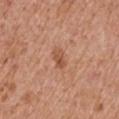The lesion was photographed on a routine skin check and not biopsied; there is no pathology result.
Approximately 2.5 mm at its widest.
The tile uses white-light illumination.
A male subject, approximately 65 years of age.
Located on the right upper arm.
A roughly 15 mm field-of-view crop from a total-body skin photograph.